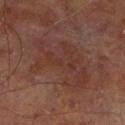The lesion was photographed on a routine skin check and not biopsied; there is no pathology result.
Cropped from a whole-body photographic skin survey; the tile spans about 15 mm.
A male subject, in their mid- to late 60s.
From the right lower leg.
The lesion's longest dimension is about 7.5 mm.